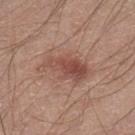Clinical impression: The lesion was tiled from a total-body skin photograph and was not biopsied. Clinical summary: A 15 mm crop from a total-body photograph taken for skin-cancer surveillance. The lesion-visualizer software estimated an area of roughly 12 mm², a shape eccentricity near 0.9, and a shape-asymmetry score of about 0.35 (0 = symmetric). It also reported about 10 CIELAB-L* units darker than the surrounding skin. It also reported an automated nevus-likeness rating near 75 out of 100. A male subject, aged approximately 20. The tile uses white-light illumination. The lesion is on the left lower leg. Longest diameter approximately 6 mm.